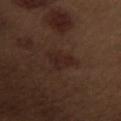biopsy_status: not biopsied; imaged during a skin examination
site: left upper arm
lighting: white-light
patient:
  sex: male
  age_approx: 70
lesion_size:
  long_diameter_mm_approx: 3.5
image:
  source: total-body photography crop
  field_of_view_mm: 15